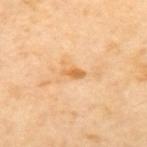Impression: Part of a total-body skin-imaging series; this lesion was reviewed on a skin check and was not flagged for biopsy. Clinical summary: A roughly 15 mm field-of-view crop from a total-body skin photograph. A male patient aged 63 to 67. On the upper back. About 2.5 mm across. Captured under cross-polarized illumination.